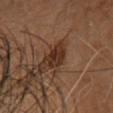workup — catalogued during a skin exam; not biopsied
acquisition — total-body-photography crop, ~15 mm field of view
subject — female, aged around 60
anatomic site — the head or neck
lesion size — about 4 mm
tile lighting — cross-polarized
automated lesion analysis — a footprint of about 5.5 mm², a shape eccentricity near 0.9, and two-axis asymmetry of about 0.2; a classifier nevus-likeness of about 80/100